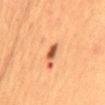Q: Was a biopsy performed?
A: imaged on a skin check; not biopsied
Q: What is the anatomic site?
A: the mid back
Q: What is the imaging modality?
A: ~15 mm tile from a whole-body skin photo
Q: Lesion size?
A: ~2.5 mm (longest diameter)
Q: Automated lesion metrics?
A: a footprint of about 2.5 mm² and a symmetry-axis asymmetry near 0.25; a lesion–skin lightness drop of about 15 and a lesion-to-skin contrast of about 10.5 (normalized; higher = more distinct); a border-irregularity index near 2.5/10, a within-lesion color-variation index near 0/10, and radial color variation of about 0; a nevus-likeness score of about 65/100
Q: Who is the patient?
A: female, aged 53 to 57